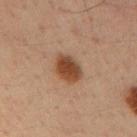Q: Was this lesion biopsied?
A: total-body-photography surveillance lesion; no biopsy
Q: How was this image acquired?
A: total-body-photography crop, ~15 mm field of view
Q: What is the lesion's diameter?
A: ~4 mm (longest diameter)
Q: Patient demographics?
A: male, approximately 35 years of age
Q: Illumination type?
A: cross-polarized
Q: Where on the body is the lesion?
A: the right upper arm